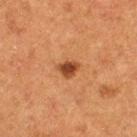Imaged during a routine full-body skin examination; the lesion was not biopsied and no histopathology is available. A female patient, in their 50s. Imaged with cross-polarized lighting. On the left thigh. This image is a 15 mm lesion crop taken from a total-body photograph. Automated tile analysis of the lesion measured a lesion color around L≈37 a*≈23 b*≈32 in CIELAB and a lesion–skin lightness drop of about 12. The analysis additionally found a border-irregularity rating of about 2.5/10 and a color-variation rating of about 2/10. And it measured a classifier nevus-likeness of about 95/100. Longest diameter approximately 2.5 mm.